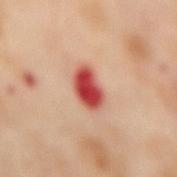Assessment: Recorded during total-body skin imaging; not selected for excision or biopsy. Background: A female patient in their mid- to late 50s. The tile uses cross-polarized illumination. A lesion tile, about 15 mm wide, cut from a 3D total-body photograph. Longest diameter approximately 4 mm. On the mid back.